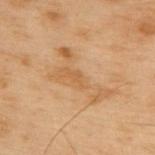The lesion was photographed on a routine skin check and not biopsied; there is no pathology result.
The lesion is on the back.
A 15 mm close-up extracted from a 3D total-body photography capture.
A male subject aged around 55.